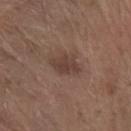biopsy_status: not biopsied; imaged during a skin examination
automated_metrics:
  cielab_L: 40
  cielab_a: 17
  cielab_b: 23
  vs_skin_darker_L: 8.0
  vs_skin_contrast_norm: 7.0
  border_irregularity_0_10: 2.5
  color_variation_0_10: 2.0
  peripheral_color_asymmetry: 0.5
lesion_size:
  long_diameter_mm_approx: 3.5
lighting: white-light
patient:
  sex: male
  age_approx: 65
image:
  source: total-body photography crop
  field_of_view_mm: 15
site: left forearm